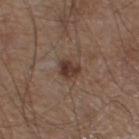Imaged during a routine full-body skin examination; the lesion was not biopsied and no histopathology is available.
A male subject, aged around 55.
Located on the left lower leg.
A close-up tile cropped from a whole-body skin photograph, about 15 mm across.